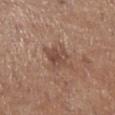Part of a total-body skin-imaging series; this lesion was reviewed on a skin check and was not flagged for biopsy. The lesion's longest dimension is about 3 mm. The subject is a male in their mid-70s. Imaged with white-light lighting. A 15 mm crop from a total-body photograph taken for skin-cancer surveillance. The lesion is on the right lower leg.